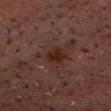- notes — catalogued during a skin exam; not biopsied